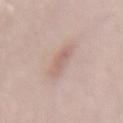On the mid back.
The lesion's longest dimension is about 4 mm.
This image is a 15 mm lesion crop taken from a total-body photograph.
An algorithmic analysis of the crop reported a footprint of about 6.5 mm², a shape eccentricity near 0.9, and a shape-asymmetry score of about 0.2 (0 = symmetric).
The patient is a female aged 48–52.
This is a white-light tile.